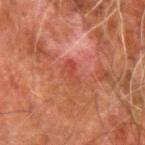Clinical impression: Captured during whole-body skin photography for melanoma surveillance; the lesion was not biopsied. Clinical summary: On the left forearm. The lesion-visualizer software estimated roughly 6 lightness units darker than nearby skin and a normalized lesion–skin contrast near 5. The analysis additionally found a within-lesion color-variation index near 1/10 and peripheral color asymmetry of about 0.5. This image is a 15 mm lesion crop taken from a total-body photograph. A male subject aged 78–82.